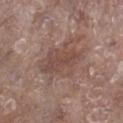Q: Is there a histopathology result?
A: no biopsy performed (imaged during a skin exam)
Q: Who is the patient?
A: female, aged approximately 75
Q: What is the imaging modality?
A: 15 mm crop, total-body photography
Q: Lesion location?
A: the right lower leg